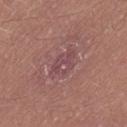| field | value |
|---|---|
| biopsy status | total-body-photography surveillance lesion; no biopsy |
| lesion size | ~4 mm (longest diameter) |
| acquisition | ~15 mm crop, total-body skin-cancer survey |
| subject | male, about 25 years old |
| lighting | white-light illumination |
| site | the lower back |
| automated metrics | an automated nevus-likeness rating near 0 out of 100 |